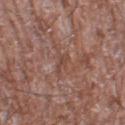Imaged during a routine full-body skin examination; the lesion was not biopsied and no histopathology is available. Longest diameter approximately 2.5 mm. A 15 mm close-up extracted from a 3D total-body photography capture. The lesion is located on the left lower leg. Captured under white-light illumination. A male patient, aged around 60.